Longest diameter approximately 2 mm. A female subject, approximately 60 years of age. The tile uses cross-polarized illumination. Located on the upper back. A 15 mm close-up tile from a total-body photography series done for melanoma screening.
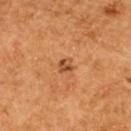The biopsy diagnosis was an atypical melanocytic neoplasm — a borderline lesion of uncertain malignant potential.